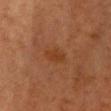notes: no biopsy performed (imaged during a skin exam)
subject: female, aged around 40
illumination: cross-polarized illumination
automated metrics: an area of roughly 4.5 mm² and an eccentricity of roughly 0.7; border irregularity of about 2 on a 0–10 scale, internal color variation of about 1 on a 0–10 scale, and a peripheral color-asymmetry measure near 0.5; lesion-presence confidence of about 100/100
lesion size: about 3 mm
anatomic site: the chest
acquisition: ~15 mm crop, total-body skin-cancer survey